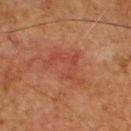Imaged with cross-polarized lighting.
Automated tile analysis of the lesion measured an area of roughly 9.5 mm², an outline eccentricity of about 0.75 (0 = round, 1 = elongated), and a symmetry-axis asymmetry near 0.65. And it measured a lesion color around L≈43 a*≈28 b*≈31 in CIELAB, about 6 CIELAB-L* units darker than the surrounding skin, and a lesion-to-skin contrast of about 4.5 (normalized; higher = more distinct). The software also gave a border-irregularity rating of about 10/10, a color-variation rating of about 1.5/10, and peripheral color asymmetry of about 0.5.
The lesion is located on the chest.
A 15 mm crop from a total-body photograph taken for skin-cancer surveillance.
The patient is a male in their mid- to late 50s.